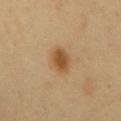biopsy status = imaged on a skin check; not biopsied
lighting = cross-polarized illumination
subject = male, roughly 50 years of age
image source = 15 mm crop, total-body photography
size = about 3.5 mm
automated metrics = an area of roughly 6 mm², a shape eccentricity near 0.7, and a symmetry-axis asymmetry near 0.15
body site = the chest